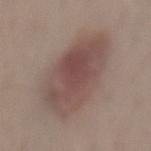The lesion was tiled from a total-body skin photograph and was not biopsied. From the lower back. Cropped from a total-body skin-imaging series; the visible field is about 15 mm. The tile uses white-light illumination. The patient is a female aged approximately 45.